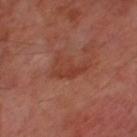{
  "biopsy_status": "not biopsied; imaged during a skin examination",
  "site": "left thigh",
  "lesion_size": {
    "long_diameter_mm_approx": 4.0
  },
  "patient": {
    "sex": "male",
    "age_approx": 70
  },
  "lighting": "cross-polarized",
  "image": {
    "source": "total-body photography crop",
    "field_of_view_mm": 15
  }
}